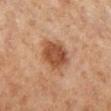  biopsy_status: not biopsied; imaged during a skin examination
  patient:
    sex: male
    age_approx: 65
  site: left lower leg
  lighting: cross-polarized
  automated_metrics:
    area_mm2_approx: 13.0
    shape_asymmetry: 0.2
    cielab_L: 40
    cielab_a: 19
    cielab_b: 28
    vs_skin_darker_L: 10.0
    vs_skin_contrast_norm: 8.5
    nevus_likeness_0_100: 90
  image:
    source: total-body photography crop
    field_of_view_mm: 15
  lesion_size:
    long_diameter_mm_approx: 4.0